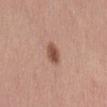Captured during whole-body skin photography for melanoma surveillance; the lesion was not biopsied.
A lesion tile, about 15 mm wide, cut from a 3D total-body photograph.
A female subject in their mid-50s.
This is a white-light tile.
Longest diameter approximately 3 mm.
The total-body-photography lesion software estimated an average lesion color of about L≈52 a*≈23 b*≈28 (CIELAB) and about 13 CIELAB-L* units darker than the surrounding skin. It also reported a detector confidence of about 100 out of 100 that the crop contains a lesion.
The lesion is located on the abdomen.